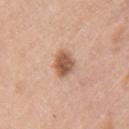notes = no biopsy performed (imaged during a skin exam) | tile lighting = white-light illumination | patient = female, roughly 60 years of age | size = ≈3 mm | site = the arm | image source = ~15 mm tile from a whole-body skin photo.